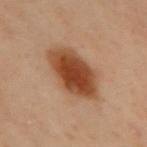This lesion was catalogued during total-body skin photography and was not selected for biopsy.
About 7 mm across.
The lesion is on the upper back.
A region of skin cropped from a whole-body photographic capture, roughly 15 mm wide.
The patient is a female aged 58–62.
Automated image analysis of the tile measured an outline eccentricity of about 0.85 (0 = round, 1 = elongated) and a symmetry-axis asymmetry near 0.15. The software also gave roughly 13 lightness units darker than nearby skin and a normalized border contrast of about 11.5. It also reported a border-irregularity rating of about 2/10 and a color-variation rating of about 5/10.
The tile uses cross-polarized illumination.